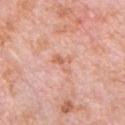Clinical impression: The lesion was photographed on a routine skin check and not biopsied; there is no pathology result. Context: The recorded lesion diameter is about 2.5 mm. An algorithmic analysis of the crop reported an area of roughly 4 mm² and a symmetry-axis asymmetry near 0.5. The software also gave an average lesion color of about L≈65 a*≈26 b*≈32 (CIELAB), roughly 8 lightness units darker than nearby skin, and a normalized lesion–skin contrast near 5.5. The software also gave a border-irregularity index near 6/10 and internal color variation of about 0.5 on a 0–10 scale. The analysis additionally found a classifier nevus-likeness of about 0/100. The tile uses white-light illumination. From the front of the torso. The subject is a male approximately 80 years of age. This image is a 15 mm lesion crop taken from a total-body photograph.